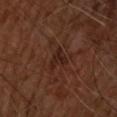Captured during whole-body skin photography for melanoma surveillance; the lesion was not biopsied.